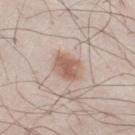This lesion was catalogued during total-body skin photography and was not selected for biopsy. The lesion is on the leg. The patient is a male in their mid- to late 20s. A lesion tile, about 15 mm wide, cut from a 3D total-body photograph.